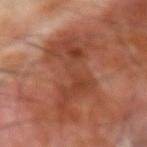Part of a total-body skin-imaging series; this lesion was reviewed on a skin check and was not flagged for biopsy. From the right forearm. Longest diameter approximately 9 mm. Imaged with cross-polarized lighting. The subject is a male aged around 70. Automated tile analysis of the lesion measured a shape eccentricity near 0.8 and two-axis asymmetry of about 0.35. And it measured a border-irregularity index near 7.5/10. The software also gave a classifier nevus-likeness of about 0/100 and a detector confidence of about 100 out of 100 that the crop contains a lesion. A roughly 15 mm field-of-view crop from a total-body skin photograph.